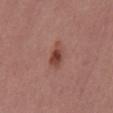The lesion is located on the chest. The recorded lesion diameter is about 4 mm. Automated image analysis of the tile measured an average lesion color of about L≈44 a*≈25 b*≈26 (CIELAB), a lesion–skin lightness drop of about 11, and a normalized border contrast of about 8.5. The software also gave a border-irregularity index near 3.5/10 and internal color variation of about 2.5 on a 0–10 scale. It also reported an automated nevus-likeness rating near 100 out of 100 and a lesion-detection confidence of about 100/100. This is a white-light tile. A 15 mm crop from a total-body photograph taken for skin-cancer surveillance. A male subject roughly 55 years of age.